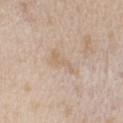notes: total-body-photography surveillance lesion; no biopsy
diameter: ~3.5 mm (longest diameter)
imaging modality: total-body-photography crop, ~15 mm field of view
tile lighting: white-light illumination
location: the right upper arm
patient: male, in their mid- to late 40s
TBP lesion metrics: an area of roughly 3.5 mm², a shape eccentricity near 0.95, and a shape-asymmetry score of about 0.55 (0 = symmetric); about 6 CIELAB-L* units darker than the surrounding skin and a lesion-to-skin contrast of about 5 (normalized; higher = more distinct); border irregularity of about 7 on a 0–10 scale, a color-variation rating of about 0/10, and radial color variation of about 0; an automated nevus-likeness rating near 0 out of 100 and lesion-presence confidence of about 100/100